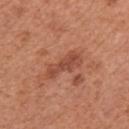| key | value |
|---|---|
| biopsy status | no biopsy performed (imaged during a skin exam) |
| location | the right upper arm |
| patient | female, approximately 50 years of age |
| image | total-body-photography crop, ~15 mm field of view |
| tile lighting | white-light |